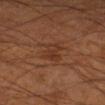Captured during whole-body skin photography for melanoma surveillance; the lesion was not biopsied. A lesion tile, about 15 mm wide, cut from a 3D total-body photograph. A male subject, aged 53–57. From the right lower leg. The total-body-photography lesion software estimated a lesion color around L≈31 a*≈20 b*≈27 in CIELAB, roughly 5 lightness units darker than nearby skin, and a normalized border contrast of about 5. It also reported a within-lesion color-variation index near 2.5/10 and a peripheral color-asymmetry measure near 1. The analysis additionally found a nevus-likeness score of about 0/100. Captured under cross-polarized illumination. The lesion's longest dimension is about 3 mm.